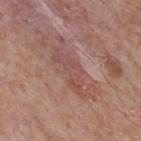This lesion was catalogued during total-body skin photography and was not selected for biopsy. Captured under white-light illumination. A lesion tile, about 15 mm wide, cut from a 3D total-body photograph. Located on the back. About 5 mm across. A male subject, about 70 years old.